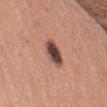{
  "biopsy_status": "not biopsied; imaged during a skin examination",
  "patient": {
    "sex": "female",
    "age_approx": 55
  },
  "lesion_size": {
    "long_diameter_mm_approx": 3.5
  },
  "image": {
    "source": "total-body photography crop",
    "field_of_view_mm": 15
  },
  "site": "left forearm",
  "lighting": "white-light"
}